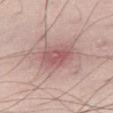Assessment: Imaged during a routine full-body skin examination; the lesion was not biopsied and no histopathology is available. Context: The recorded lesion diameter is about 3.5 mm. This image is a 15 mm lesion crop taken from a total-body photograph. Captured under white-light illumination. The patient is a male roughly 35 years of age. On the leg.